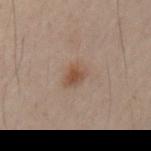{"biopsy_status": "not biopsied; imaged during a skin examination", "automated_metrics": {"area_mm2_approx": 5.0, "eccentricity": 0.7, "shape_asymmetry": 0.25, "border_irregularity_0_10": 2.0}, "site": "arm", "patient": {"sex": "male", "age_approx": 50}, "image": {"source": "total-body photography crop", "field_of_view_mm": 15}, "lesion_size": {"long_diameter_mm_approx": 3.0}, "lighting": "cross-polarized"}